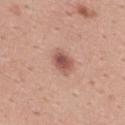The lesion is on the back. A region of skin cropped from a whole-body photographic capture, roughly 15 mm wide. The subject is a male aged 23–27.